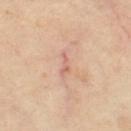Findings:
- biopsy status · catalogued during a skin exam; not biopsied
- acquisition · total-body-photography crop, ~15 mm field of view
- location · the left thigh
- patient · female, about 50 years old